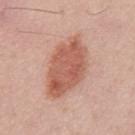Q: Lesion location?
A: the mid back
Q: Who is the patient?
A: male, aged 48 to 52
Q: Lesion size?
A: ~8 mm (longest diameter)
Q: What is the imaging modality?
A: total-body-photography crop, ~15 mm field of view
Q: What did automated image analysis measure?
A: an area of roughly 24 mm² and a shape eccentricity near 0.85; border irregularity of about 2 on a 0–10 scale, internal color variation of about 4 on a 0–10 scale, and a peripheral color-asymmetry measure near 1.5
Q: What lighting was used for the tile?
A: white-light illumination